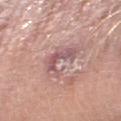Q: Was a biopsy performed?
A: no biopsy performed (imaged during a skin exam)
Q: Lesion size?
A: about 4.5 mm
Q: Who is the patient?
A: male, roughly 60 years of age
Q: How was this image acquired?
A: ~15 mm tile from a whole-body skin photo
Q: What did automated image analysis measure?
A: an average lesion color of about L≈56 a*≈22 b*≈19 (CIELAB), about 10 CIELAB-L* units darker than the surrounding skin, and a normalized lesion–skin contrast near 7.5
Q: What lighting was used for the tile?
A: white-light
Q: Where on the body is the lesion?
A: the right forearm